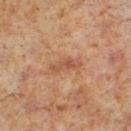biopsy status: total-body-photography surveillance lesion; no biopsy
TBP lesion metrics: a lesion area of about 4 mm² and a shape-asymmetry score of about 0.45 (0 = symmetric); a lesion–skin lightness drop of about 8 and a normalized lesion–skin contrast near 6
illumination: cross-polarized
patient: male, approximately 60 years of age
imaging modality: 15 mm crop, total-body photography
location: the left lower leg
diameter: ~4 mm (longest diameter)